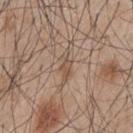On the mid back.
A male patient aged 43–47.
A region of skin cropped from a whole-body photographic capture, roughly 15 mm wide.
An algorithmic analysis of the crop reported a lesion area of about 3.5 mm² and an outline eccentricity of about 0.9 (0 = round, 1 = elongated). It also reported a border-irregularity index near 5/10 and a color-variation rating of about 1.5/10. The analysis additionally found a classifier nevus-likeness of about 0/100 and a lesion-detection confidence of about 85/100.
Longest diameter approximately 3.5 mm.
Imaged with white-light lighting.